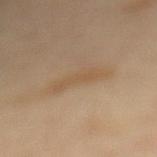Q: Patient demographics?
A: female, approximately 65 years of age
Q: How was this image acquired?
A: ~15 mm tile from a whole-body skin photo
Q: What is the anatomic site?
A: the mid back
Q: Lesion size?
A: ~5 mm (longest diameter)
Q: What lighting was used for the tile?
A: cross-polarized illumination